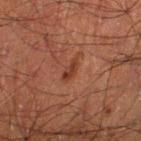Clinical impression: Part of a total-body skin-imaging series; this lesion was reviewed on a skin check and was not flagged for biopsy. Acquisition and patient details: The lesion is located on the left thigh. Automated image analysis of the tile measured an area of roughly 3 mm² and a shape-asymmetry score of about 0.35 (0 = symmetric). And it measured a classifier nevus-likeness of about 45/100. Captured under cross-polarized illumination. The subject is a male about 50 years old. The lesion's longest dimension is about 3 mm. This image is a 15 mm lesion crop taken from a total-body photograph.